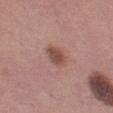lesion size: ≈3 mm
imaging modality: 15 mm crop, total-body photography
patient: female, aged around 50
lighting: white-light illumination
body site: the leg